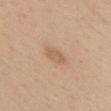<lesion>
  <biopsy_status>not biopsied; imaged during a skin examination</biopsy_status>
  <image>
    <source>total-body photography crop</source>
    <field_of_view_mm>15</field_of_view_mm>
  </image>
  <lesion_size>
    <long_diameter_mm_approx>3.5</long_diameter_mm_approx>
  </lesion_size>
  <lighting>white-light</lighting>
  <site>head or neck</site>
  <patient>
    <sex>female</sex>
    <age_approx>45</age_approx>
  </patient>
</lesion>